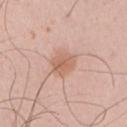Q: Was a biopsy performed?
A: total-body-photography surveillance lesion; no biopsy
Q: How was this image acquired?
A: 15 mm crop, total-body photography
Q: What did automated image analysis measure?
A: a lesion area of about 6.5 mm², a shape eccentricity near 0.5, and a symmetry-axis asymmetry near 0.2; a classifier nevus-likeness of about 30/100 and lesion-presence confidence of about 100/100
Q: How was the tile lit?
A: white-light
Q: What is the anatomic site?
A: the right upper arm
Q: What are the patient's age and sex?
A: male, roughly 40 years of age
Q: Lesion size?
A: ~3 mm (longest diameter)